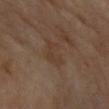  biopsy_status: not biopsied; imaged during a skin examination
  site: arm
  lesion_size:
    long_diameter_mm_approx: 3.0
  image:
    source: total-body photography crop
    field_of_view_mm: 15
  patient:
    sex: female
    age_approx: 70
  automated_metrics:
    area_mm2_approx: 6.0
    eccentricity: 0.75
    shape_asymmetry: 0.35
    border_irregularity_0_10: 4.5
    color_variation_0_10: 1.0
    peripheral_color_asymmetry: 0.5
  lighting: cross-polarized